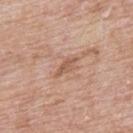Q: Was this lesion biopsied?
A: total-body-photography surveillance lesion; no biopsy
Q: What are the patient's age and sex?
A: male, aged 63 to 67
Q: Lesion location?
A: the upper back
Q: What lighting was used for the tile?
A: white-light
Q: What kind of image is this?
A: ~15 mm crop, total-body skin-cancer survey
Q: What did automated image analysis measure?
A: a footprint of about 3.5 mm², an outline eccentricity of about 0.9 (0 = round, 1 = elongated), and a shape-asymmetry score of about 0.3 (0 = symmetric); a lesion color around L≈56 a*≈21 b*≈30 in CIELAB and roughly 9 lightness units darker than nearby skin; border irregularity of about 3.5 on a 0–10 scale and radial color variation of about 0.5; a nevus-likeness score of about 0/100 and lesion-presence confidence of about 95/100